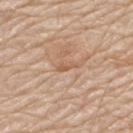The lesion was photographed on a routine skin check and not biopsied; there is no pathology result. The lesion-visualizer software estimated a lesion area of about 2 mm² and a shape eccentricity near 0.9. The analysis additionally found a lesion color around L≈58 a*≈20 b*≈33 in CIELAB, about 8 CIELAB-L* units darker than the surrounding skin, and a normalized border contrast of about 5.5. The analysis additionally found a classifier nevus-likeness of about 0/100 and a lesion-detection confidence of about 60/100. A 15 mm close-up tile from a total-body photography series done for melanoma screening. From the upper back. Imaged with white-light lighting. A male patient aged approximately 80.